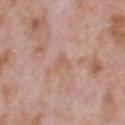Q: Is there a histopathology result?
A: imaged on a skin check; not biopsied
Q: Automated lesion metrics?
A: a border-irregularity index near 4.5/10; a nevus-likeness score of about 0/100 and a detector confidence of about 100 out of 100 that the crop contains a lesion
Q: Where on the body is the lesion?
A: the head or neck
Q: What is the imaging modality?
A: ~15 mm crop, total-body skin-cancer survey
Q: What is the lesion's diameter?
A: ~2.5 mm (longest diameter)
Q: Patient demographics?
A: male, approximately 70 years of age
Q: What lighting was used for the tile?
A: white-light illumination